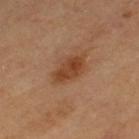{"biopsy_status": "not biopsied; imaged during a skin examination", "image": {"source": "total-body photography crop", "field_of_view_mm": 15}, "lesion_size": {"long_diameter_mm_approx": 5.0}, "lighting": "cross-polarized", "patient": {"sex": "female", "age_approx": 60}, "site": "left thigh"}